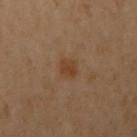This lesion was catalogued during total-body skin photography and was not selected for biopsy.
This is a cross-polarized tile.
A female patient, aged 58–62.
From the upper back.
Cropped from a whole-body photographic skin survey; the tile spans about 15 mm.